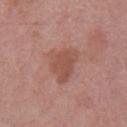{
  "biopsy_status": "not biopsied; imaged during a skin examination",
  "patient": {
    "sex": "male",
    "age_approx": 55
  },
  "image": {
    "source": "total-body photography crop",
    "field_of_view_mm": 15
  },
  "lighting": "white-light",
  "site": "right lower leg",
  "automated_metrics": {
    "cielab_L": 51,
    "cielab_a": 23,
    "cielab_b": 27,
    "vs_skin_contrast_norm": 6.0
  }
}